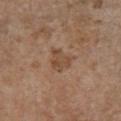follow-up=catalogued during a skin exam; not biopsied | patient=female, about 65 years old | anatomic site=the chest | imaging modality=~15 mm tile from a whole-body skin photo.